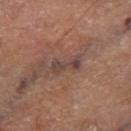Imaged during a routine full-body skin examination; the lesion was not biopsied and no histopathology is available.
On the right thigh.
The patient is a male aged approximately 70.
The recorded lesion diameter is about 3.5 mm.
The tile uses cross-polarized illumination.
A region of skin cropped from a whole-body photographic capture, roughly 15 mm wide.